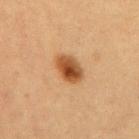Impression: Recorded during total-body skin imaging; not selected for excision or biopsy. Background: The lesion is on the back. Automated image analysis of the tile measured a normalized lesion–skin contrast near 10.5. It also reported a border-irregularity index near 1/10 and peripheral color asymmetry of about 2. Longest diameter approximately 3.5 mm. The tile uses cross-polarized illumination. The patient is a female in their 40s. A region of skin cropped from a whole-body photographic capture, roughly 15 mm wide.